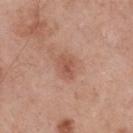Recorded during total-body skin imaging; not selected for excision or biopsy. This is a white-light tile. The lesion is located on the upper back. A male subject, approximately 55 years of age. Measured at roughly 3 mm in maximum diameter. Cropped from a total-body skin-imaging series; the visible field is about 15 mm.